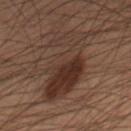<lesion>
  <biopsy_status>not biopsied; imaged during a skin examination</biopsy_status>
  <lighting>cross-polarized</lighting>
  <lesion_size>
    <long_diameter_mm_approx>8.0</long_diameter_mm_approx>
  </lesion_size>
  <patient>
    <sex>male</sex>
    <age_approx>55</age_approx>
  </patient>
  <automated_metrics>
    <area_mm2_approx>27.0</area_mm2_approx>
    <eccentricity>0.85</eccentricity>
    <shape_asymmetry>0.5</shape_asymmetry>
    <border_irregularity_0_10>7.0</border_irregularity_0_10>
    <color_variation_0_10>6.5</color_variation_0_10>
    <peripheral_color_asymmetry>2.5</peripheral_color_asymmetry>
    <nevus_likeness_0_100>55</nevus_likeness_0_100>
    <lesion_detection_confidence_0_100>100</lesion_detection_confidence_0_100>
  </automated_metrics>
  <site>right thigh</site>
  <image>
    <source>total-body photography crop</source>
    <field_of_view_mm>15</field_of_view_mm>
  </image>
</lesion>